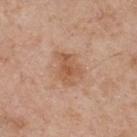{"biopsy_status": "not biopsied; imaged during a skin examination", "image": {"source": "total-body photography crop", "field_of_view_mm": 15}, "patient": {"sex": "male", "age_approx": 55}, "site": "upper back", "lighting": "white-light"}